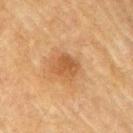Clinical impression:
Part of a total-body skin-imaging series; this lesion was reviewed on a skin check and was not flagged for biopsy.
Image and clinical context:
The total-body-photography lesion software estimated an area of roughly 6 mm², an eccentricity of roughly 0.2, and a symmetry-axis asymmetry near 0.3. It also reported a border-irregularity rating of about 2.5/10 and a color-variation rating of about 2.5/10. Imaged with cross-polarized lighting. A region of skin cropped from a whole-body photographic capture, roughly 15 mm wide. A male patient aged around 75. From the right forearm.